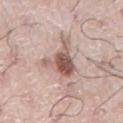image: total-body-photography crop, ~15 mm field of view
anatomic site: the abdomen
TBP lesion metrics: an area of roughly 11 mm², an outline eccentricity of about 0.6 (0 = round, 1 = elongated), and a shape-asymmetry score of about 0.6 (0 = symmetric); an average lesion color of about L≈56 a*≈18 b*≈23 (CIELAB), roughly 14 lightness units darker than nearby skin, and a lesion-to-skin contrast of about 9.5 (normalized; higher = more distinct); a nevus-likeness score of about 85/100 and a detector confidence of about 100 out of 100 that the crop contains a lesion
size: ~5 mm (longest diameter)
subject: male, in their mid- to late 70s